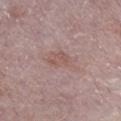  biopsy_status: not biopsied; imaged during a skin examination
  automated_metrics:
    border_irregularity_0_10: 3.5
    color_variation_0_10: 2.5
    peripheral_color_asymmetry: 1.0
  patient:
    sex: male
    age_approx: 75
  site: right lower leg
  image:
    source: total-body photography crop
    field_of_view_mm: 15